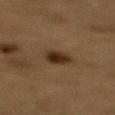The recorded lesion diameter is about 2.5 mm.
The lesion-visualizer software estimated a mean CIELAB color near L≈25 a*≈14 b*≈24 and a lesion–skin lightness drop of about 10. It also reported border irregularity of about 2 on a 0–10 scale and a color-variation rating of about 4/10.
Imaged with cross-polarized lighting.
A male patient, aged approximately 85 or older.
On the back.
A close-up tile cropped from a whole-body skin photograph, about 15 mm across.